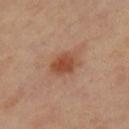Findings:
• notes — imaged on a skin check; not biopsied
• image — total-body-photography crop, ~15 mm field of view
• size — about 3.5 mm
• lighting — cross-polarized illumination
• automated metrics — a nevus-likeness score of about 95/100 and a detector confidence of about 100 out of 100 that the crop contains a lesion
• anatomic site — the right thigh
• subject — female, aged around 45